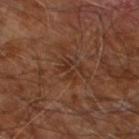The lesion was tiled from a total-body skin photograph and was not biopsied. Approximately 2.5 mm at its widest. The patient is a male roughly 60 years of age. The tile uses cross-polarized illumination. Automated image analysis of the tile measured a footprint of about 3 mm², an outline eccentricity of about 0.8 (0 = round, 1 = elongated), and two-axis asymmetry of about 0.35. It also reported a mean CIELAB color near L≈30 a*≈19 b*≈26, about 5 CIELAB-L* units darker than the surrounding skin, and a normalized border contrast of about 5.5. The software also gave a border-irregularity index near 4.5/10, a within-lesion color-variation index near 0.5/10, and a peripheral color-asymmetry measure near 0. The analysis additionally found an automated nevus-likeness rating near 0 out of 100. The lesion is located on the right leg. Cropped from a whole-body photographic skin survey; the tile spans about 15 mm.